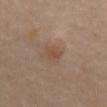Impression:
Captured during whole-body skin photography for melanoma surveillance; the lesion was not biopsied.
Context:
Cropped from a whole-body photographic skin survey; the tile spans about 15 mm. Automated tile analysis of the lesion measured an area of roughly 2.5 mm² and a shape eccentricity near 0.75. The analysis additionally found a mean CIELAB color near L≈48 a*≈19 b*≈28 and about 7 CIELAB-L* units darker than the surrounding skin. The lesion is on the mid back. A female subject roughly 55 years of age.